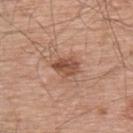Part of a total-body skin-imaging series; this lesion was reviewed on a skin check and was not flagged for biopsy.
Imaged with white-light lighting.
Automated tile analysis of the lesion measured an area of roughly 6 mm² and an outline eccentricity of about 0.7 (0 = round, 1 = elongated). The software also gave a mean CIELAB color near L≈51 a*≈23 b*≈30, about 11 CIELAB-L* units darker than the surrounding skin, and a normalized border contrast of about 8. The software also gave border irregularity of about 2.5 on a 0–10 scale, internal color variation of about 4.5 on a 0–10 scale, and radial color variation of about 1.5.
The lesion's longest dimension is about 3.5 mm.
This image is a 15 mm lesion crop taken from a total-body photograph.
The lesion is located on the back.
A male patient, approximately 55 years of age.